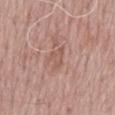Part of a total-body skin-imaging series; this lesion was reviewed on a skin check and was not flagged for biopsy.
A male subject, roughly 65 years of age.
The total-body-photography lesion software estimated a lesion color around L≈55 a*≈21 b*≈25 in CIELAB. It also reported an automated nevus-likeness rating near 0 out of 100 and lesion-presence confidence of about 100/100.
A 15 mm close-up extracted from a 3D total-body photography capture.
Located on the back.
Captured under white-light illumination.